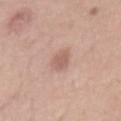Clinical summary: A 15 mm crop from a total-body photograph taken for skin-cancer surveillance. Located on the mid back. A male patient about 30 years old. The lesion's longest dimension is about 3.5 mm.